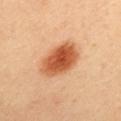biopsy_status: not biopsied; imaged during a skin examination